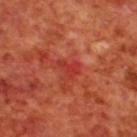Clinical impression:
The lesion was photographed on a routine skin check and not biopsied; there is no pathology result.
Clinical summary:
The lesion is located on the upper back. The subject is a male aged 68 to 72. Longest diameter approximately 2.5 mm. Automated image analysis of the tile measured a footprint of about 3 mm², an outline eccentricity of about 0.8 (0 = round, 1 = elongated), and a symmetry-axis asymmetry near 0.5. The software also gave a mean CIELAB color near L≈39 a*≈40 b*≈34, about 6 CIELAB-L* units darker than the surrounding skin, and a normalized lesion–skin contrast near 5. The analysis additionally found lesion-presence confidence of about 95/100. A close-up tile cropped from a whole-body skin photograph, about 15 mm across. This is a cross-polarized tile.